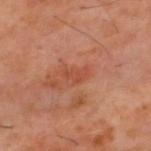notes = imaged on a skin check; not biopsied | site = the upper back | image = total-body-photography crop, ~15 mm field of view | size = ~2.5 mm (longest diameter) | subject = male, approximately 60 years of age | tile lighting = cross-polarized.